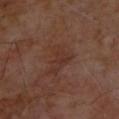image-analysis metrics: a mean CIELAB color near L≈31 a*≈19 b*≈24, a lesion–skin lightness drop of about 5, and a lesion-to-skin contrast of about 5 (normalized; higher = more distinct); a classifier nevus-likeness of about 0/100 and a detector confidence of about 100 out of 100 that the crop contains a lesion | tile lighting: cross-polarized illumination | image: ~15 mm tile from a whole-body skin photo | size: ~3.5 mm (longest diameter) | patient: male, about 70 years old | site: the upper back.